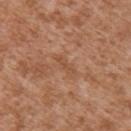Part of a total-body skin-imaging series; this lesion was reviewed on a skin check and was not flagged for biopsy. The patient is a male in their mid- to late 40s. On the right upper arm. A region of skin cropped from a whole-body photographic capture, roughly 15 mm wide. Automated tile analysis of the lesion measured a lesion area of about 2 mm², an outline eccentricity of about 0.95 (0 = round, 1 = elongated), and a symmetry-axis asymmetry near 0.5. It also reported roughly 7 lightness units darker than nearby skin and a normalized lesion–skin contrast near 5. Approximately 3 mm at its widest.